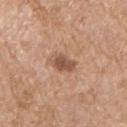The lesion was tiled from a total-body skin photograph and was not biopsied.